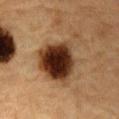Findings:
* notes · catalogued during a skin exam; not biopsied
* body site · the abdomen
* diameter · ≈7.5 mm
* lighting · cross-polarized
* image source · ~15 mm tile from a whole-body skin photo
* subject · male, approximately 85 years of age or older